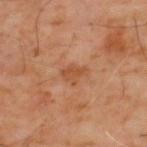notes — total-body-photography surveillance lesion; no biopsy
size — about 3 mm
subject — male, aged around 60
body site — the upper back
tile lighting — cross-polarized
acquisition — total-body-photography crop, ~15 mm field of view